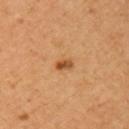| key | value |
|---|---|
| biopsy status | catalogued during a skin exam; not biopsied |
| patient | female, in their mid- to late 30s |
| image source | ~15 mm tile from a whole-body skin photo |
| illumination | cross-polarized |
| site | the left upper arm |
| image-analysis metrics | an area of roughly 2.5 mm², an eccentricity of roughly 0.65, and a symmetry-axis asymmetry near 0.2; an average lesion color of about L≈51 a*≈25 b*≈41 (CIELAB), about 12 CIELAB-L* units darker than the surrounding skin, and a normalized lesion–skin contrast near 8.5; a border-irregularity index near 1.5/10, internal color variation of about 4 on a 0–10 scale, and a peripheral color-asymmetry measure near 1.5; a classifier nevus-likeness of about 90/100 and a detector confidence of about 100 out of 100 that the crop contains a lesion |